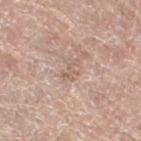The lesion was photographed on a routine skin check and not biopsied; there is no pathology result. Located on the left lower leg. A female subject, aged 58 to 62. Captured under white-light illumination. A 15 mm close-up extracted from a 3D total-body photography capture. The recorded lesion diameter is about 2.5 mm.